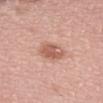Imaged during a routine full-body skin examination; the lesion was not biopsied and no histopathology is available.
The lesion is located on the left forearm.
The patient is a female in their 50s.
A 15 mm close-up extracted from a 3D total-body photography capture.